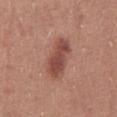This lesion was catalogued during total-body skin photography and was not selected for biopsy.
A 15 mm crop from a total-body photograph taken for skin-cancer surveillance.
This is a white-light tile.
Automated image analysis of the tile measured an area of roughly 10 mm². The analysis additionally found an average lesion color of about L≈47 a*≈25 b*≈26 (CIELAB), a lesion–skin lightness drop of about 12, and a normalized border contrast of about 8.5. And it measured a border-irregularity rating of about 3/10, a within-lesion color-variation index near 2.5/10, and peripheral color asymmetry of about 1.
A female patient, aged approximately 25.
The lesion is on the front of the torso.
Approximately 5.5 mm at its widest.